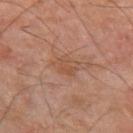Case summary:
* notes: catalogued during a skin exam; not biopsied
* size: about 3 mm
* patient: male, about 60 years old
* location: the arm
* image source: total-body-photography crop, ~15 mm field of view